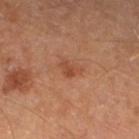The lesion was tiled from a total-body skin photograph and was not biopsied.